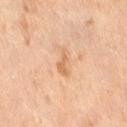  biopsy_status: not biopsied; imaged during a skin examination
  site: right thigh
  patient:
    sex: female
    age_approx: 55
  image:
    source: total-body photography crop
    field_of_view_mm: 15